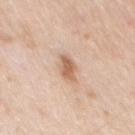<case>
<biopsy_status>not biopsied; imaged during a skin examination</biopsy_status>
<patient>
  <sex>male</sex>
  <age_approx>80</age_approx>
</patient>
<lesion_size>
  <long_diameter_mm_approx>3.0</long_diameter_mm_approx>
</lesion_size>
<image>
  <source>total-body photography crop</source>
  <field_of_view_mm>15</field_of_view_mm>
</image>
<site>chest</site>
<automated_metrics>
  <area_mm2_approx>5.0</area_mm2_approx>
  <eccentricity>0.8</eccentricity>
  <shape_asymmetry>0.25</shape_asymmetry>
  <cielab_L>63</cielab_L>
  <cielab_a>20</cielab_a>
  <cielab_b>32</cielab_b>
  <vs_skin_contrast_norm>7.5</vs_skin_contrast_norm>
</automated_metrics>
<lighting>white-light</lighting>
</case>